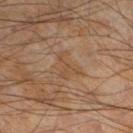notes: total-body-photography surveillance lesion; no biopsy | size: ~4 mm (longest diameter) | subject: male, about 45 years old | site: the left lower leg | image: ~15 mm crop, total-body skin-cancer survey | illumination: cross-polarized.